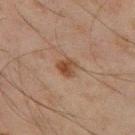Clinical summary:
Imaged with cross-polarized lighting. This image is a 15 mm lesion crop taken from a total-body photograph. A male patient, in their mid-40s. The lesion is located on the left thigh.